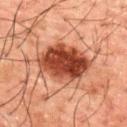Impression: Captured during whole-body skin photography for melanoma surveillance; the lesion was not biopsied. Context: The subject is a male about 50 years old. The lesion is on the upper back. This image is a 15 mm lesion crop taken from a total-body photograph.